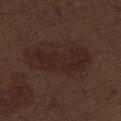follow-up = total-body-photography surveillance lesion; no biopsy | imaging modality = ~15 mm crop, total-body skin-cancer survey | diameter = about 7.5 mm | patient = male, roughly 70 years of age | TBP lesion metrics = border irregularity of about 4.5 on a 0–10 scale, a color-variation rating of about 3/10, and peripheral color asymmetry of about 1; an automated nevus-likeness rating near 65 out of 100 and a lesion-detection confidence of about 100/100 | location = the abdomen.